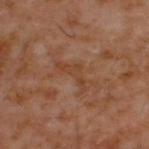Assessment: Imaged during a routine full-body skin examination; the lesion was not biopsied and no histopathology is available. Image and clinical context: A male subject, aged 58–62. The lesion is on the upper back. A lesion tile, about 15 mm wide, cut from a 3D total-body photograph.